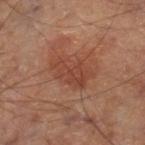Acquisition and patient details:
About 5 mm across. From the right thigh. A region of skin cropped from a whole-body photographic capture, roughly 15 mm wide.